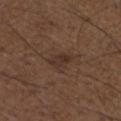workup: no biopsy performed (imaged during a skin exam)
imaging modality: ~15 mm tile from a whole-body skin photo
anatomic site: the right upper arm
patient: male, in their 50s